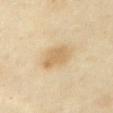Part of a total-body skin-imaging series; this lesion was reviewed on a skin check and was not flagged for biopsy. The subject is a female aged 53–57. From the front of the torso. A roughly 15 mm field-of-view crop from a total-body skin photograph. This is a cross-polarized tile.